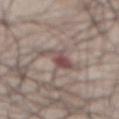Recorded during total-body skin imaging; not selected for excision or biopsy. A region of skin cropped from a whole-body photographic capture, roughly 15 mm wide. Measured at roughly 4 mm in maximum diameter. Imaged with white-light lighting. From the abdomen. The patient is a male aged approximately 50.